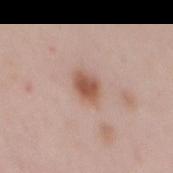follow-up — total-body-photography surveillance lesion; no biopsy | body site — the back | patient — female, roughly 40 years of age | image source — ~15 mm tile from a whole-body skin photo.